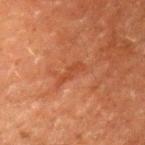acquisition: total-body-photography crop, ~15 mm field of view | tile lighting: cross-polarized | subject: male, in their 60s | lesion size: about 3.5 mm | location: the left upper arm | automated metrics: an average lesion color of about L≈37 a*≈24 b*≈31 (CIELAB), about 5 CIELAB-L* units darker than the surrounding skin, and a normalized lesion–skin contrast near 5; an automated nevus-likeness rating near 0 out of 100 and lesion-presence confidence of about 95/100.